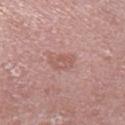• image-analysis metrics — a lesion color around L≈57 a*≈22 b*≈24 in CIELAB, about 7 CIELAB-L* units darker than the surrounding skin, and a normalized lesion–skin contrast near 5; a border-irregularity rating of about 3/10, a color-variation rating of about 2.5/10, and radial color variation of about 1; a classifier nevus-likeness of about 0/100
• subject — male, roughly 85 years of age or older
• size — ≈3 mm
• tile lighting — white-light
• anatomic site — the left lower leg
• image source — total-body-photography crop, ~15 mm field of view